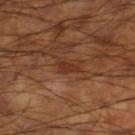{
  "biopsy_status": "not biopsied; imaged during a skin examination",
  "lighting": "cross-polarized",
  "lesion_size": {
    "long_diameter_mm_approx": 2.5
  },
  "site": "right lower leg",
  "image": {
    "source": "total-body photography crop",
    "field_of_view_mm": 15
  },
  "patient": {
    "sex": "male",
    "age_approx": 60
  }
}